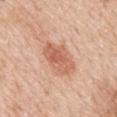Assessment:
Captured during whole-body skin photography for melanoma surveillance; the lesion was not biopsied.
Context:
Automated tile analysis of the lesion measured an area of roughly 11 mm². And it measured an automated nevus-likeness rating near 80 out of 100 and a detector confidence of about 100 out of 100 that the crop contains a lesion. The tile uses white-light illumination. Longest diameter approximately 5 mm. A lesion tile, about 15 mm wide, cut from a 3D total-body photograph. On the mid back. A male subject, roughly 60 years of age.